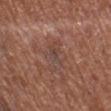<record>
<lighting>white-light</lighting>
<automated_metrics>
  <area_mm2_approx>5.5</area_mm2_approx>
  <eccentricity>0.9</eccentricity>
  <shape_asymmetry>0.4</shape_asymmetry>
  <peripheral_color_asymmetry>1.5</peripheral_color_asymmetry>
</automated_metrics>
<lesion_size>
  <long_diameter_mm_approx>4.0</long_diameter_mm_approx>
</lesion_size>
<image>
  <source>total-body photography crop</source>
  <field_of_view_mm>15</field_of_view_mm>
</image>
<site>left lower leg</site>
<patient>
  <sex>male</sex>
  <age_approx>75</age_approx>
</patient>
</record>